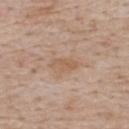Findings:
• biopsy status: no biopsy performed (imaged during a skin exam)
• body site: the back
• subject: male, roughly 75 years of age
• imaging modality: 15 mm crop, total-body photography
• lighting: white-light